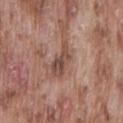Clinical impression: Part of a total-body skin-imaging series; this lesion was reviewed on a skin check and was not flagged for biopsy. Acquisition and patient details: On the lower back. The recorded lesion diameter is about 4 mm. A region of skin cropped from a whole-body photographic capture, roughly 15 mm wide. The patient is a male in their mid-70s.